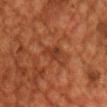{"biopsy_status": "not biopsied; imaged during a skin examination", "image": {"source": "total-body photography crop", "field_of_view_mm": 15}, "site": "chest", "patient": {"sex": "male", "age_approx": 60}}